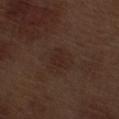notes — imaged on a skin check; not biopsied
body site — the right thigh
automated metrics — a footprint of about 3.5 mm², an outline eccentricity of about 0.65 (0 = round, 1 = elongated), and a symmetry-axis asymmetry near 0.55; about 4 CIELAB-L* units darker than the surrounding skin and a lesion-to-skin contrast of about 5 (normalized; higher = more distinct); a border-irregularity rating of about 5/10 and peripheral color asymmetry of about 0.5; lesion-presence confidence of about 100/100
tile lighting — white-light illumination
lesion diameter — ~2.5 mm (longest diameter)
subject — male, aged 68 to 72
image — ~15 mm crop, total-body skin-cancer survey